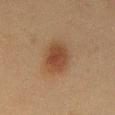The lesion was tiled from a total-body skin photograph and was not biopsied. A region of skin cropped from a whole-body photographic capture, roughly 15 mm wide. On the chest. A male subject approximately 45 years of age. Captured under cross-polarized illumination. Approximately 5 mm at its widest.